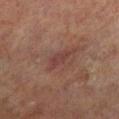Captured during whole-body skin photography for melanoma surveillance; the lesion was not biopsied. This is a cross-polarized tile. From the left lower leg. The subject is a male aged around 70. Cropped from a total-body skin-imaging series; the visible field is about 15 mm. The recorded lesion diameter is about 2.5 mm.